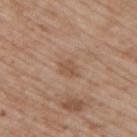  biopsy_status: not biopsied; imaged during a skin examination
  image:
    source: total-body photography crop
    field_of_view_mm: 15
  site: upper back
  lesion_size:
    long_diameter_mm_approx: 3.0
  patient:
    sex: male
    age_approx: 50